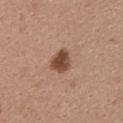follow-up: imaged on a skin check; not biopsied | patient: female, aged around 30 | site: the back | TBP lesion metrics: a footprint of about 6 mm² and two-axis asymmetry of about 0.3; a lesion color around L≈47 a*≈22 b*≈28 in CIELAB, roughly 14 lightness units darker than nearby skin, and a normalized border contrast of about 10; a border-irregularity rating of about 2.5/10, a color-variation rating of about 3.5/10, and peripheral color asymmetry of about 1 | diameter: about 3 mm | imaging modality: 15 mm crop, total-body photography | illumination: white-light illumination.